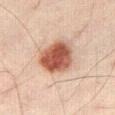Assessment: The lesion was photographed on a routine skin check and not biopsied; there is no pathology result. Clinical summary: A close-up tile cropped from a whole-body skin photograph, about 15 mm across. Captured under cross-polarized illumination. The lesion is located on the right thigh. The subject is a male aged 38 to 42.